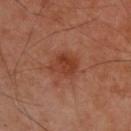{"biopsy_status": "not biopsied; imaged during a skin examination", "image": {"source": "total-body photography crop", "field_of_view_mm": 15}, "lighting": "cross-polarized", "patient": {"sex": "male", "age_approx": 50}, "site": "upper back"}